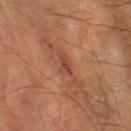notes: total-body-photography surveillance lesion; no biopsy
acquisition: 15 mm crop, total-body photography
location: the left lower leg
subject: male, aged 63 to 67